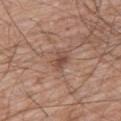  biopsy_status: not biopsied; imaged during a skin examination
  lesion_size:
    long_diameter_mm_approx: 3.0
  lighting: white-light
  site: right thigh
  patient:
    sex: male
    age_approx: 60
  image:
    source: total-body photography crop
    field_of_view_mm: 15
  automated_metrics:
    border_irregularity_0_10: 3.0
    color_variation_0_10: 3.5
    peripheral_color_asymmetry: 1.0
    nevus_likeness_0_100: 0
    lesion_detection_confidence_0_100: 100